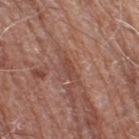The lesion was photographed on a routine skin check and not biopsied; there is no pathology result. A 15 mm crop from a total-body photograph taken for skin-cancer surveillance. An algorithmic analysis of the crop reported an area of roughly 3 mm², a shape eccentricity near 0.9, and a shape-asymmetry score of about 0.3 (0 = symmetric). The software also gave an average lesion color of about L≈45 a*≈23 b*≈27 (CIELAB). The analysis additionally found a nevus-likeness score of about 0/100 and a lesion-detection confidence of about 60/100. The lesion's longest dimension is about 2.5 mm. Imaged with white-light lighting. The subject is a male aged 78 to 82. Located on the right thigh.